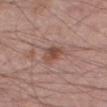The lesion was photographed on a routine skin check and not biopsied; there is no pathology result. The tile uses white-light illumination. A male subject, aged 53 to 57. From the leg. A 15 mm close-up extracted from a 3D total-body photography capture. Automated tile analysis of the lesion measured a footprint of about 5.5 mm² and a shape eccentricity near 0.7. It also reported a mean CIELAB color near L≈49 a*≈20 b*≈25 and roughly 9 lightness units darker than nearby skin. The analysis additionally found border irregularity of about 3 on a 0–10 scale, a color-variation rating of about 3.5/10, and peripheral color asymmetry of about 1. The software also gave lesion-presence confidence of about 100/100.